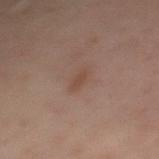Q: Was a biopsy performed?
A: imaged on a skin check; not biopsied
Q: Automated lesion metrics?
A: an area of roughly 2.5 mm², an eccentricity of roughly 0.9, and a shape-asymmetry score of about 0.25 (0 = symmetric); a lesion color around L≈35 a*≈15 b*≈21 in CIELAB and a normalized lesion–skin contrast near 6; a border-irregularity index near 3/10, a within-lesion color-variation index near 0/10, and radial color variation of about 0; an automated nevus-likeness rating near 0 out of 100 and a detector confidence of about 100 out of 100 that the crop contains a lesion
Q: What kind of image is this?
A: 15 mm crop, total-body photography
Q: Lesion location?
A: the mid back
Q: What is the lesion's diameter?
A: ~2.5 mm (longest diameter)
Q: Patient demographics?
A: male, aged approximately 40